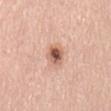| key | value |
|---|---|
| notes | imaged on a skin check; not biopsied |
| patient | male, aged 58–62 |
| tile lighting | white-light |
| image-analysis metrics | a lesion color around L≈59 a*≈23 b*≈29 in CIELAB and a normalized border contrast of about 9.5 |
| image source | ~15 mm crop, total-body skin-cancer survey |
| anatomic site | the back |